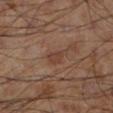Clinical impression: The lesion was tiled from a total-body skin photograph and was not biopsied. Context: The tile uses cross-polarized illumination. The patient is a male aged 58 to 62. The recorded lesion diameter is about 3 mm. Cropped from a whole-body photographic skin survey; the tile spans about 15 mm. From the left lower leg.